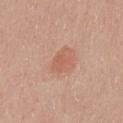– notes — catalogued during a skin exam; not biopsied
– imaging modality — ~15 mm crop, total-body skin-cancer survey
– body site — the chest
– patient — male, aged around 45